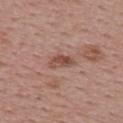{"image": {"source": "total-body photography crop", "field_of_view_mm": 15}, "lighting": "white-light", "site": "upper back", "lesion_size": {"long_diameter_mm_approx": 3.0}, "patient": {"sex": "female", "age_approx": 55}}